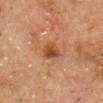This lesion was catalogued during total-body skin photography and was not selected for biopsy.
Measured at roughly 3 mm in maximum diameter.
A 15 mm crop from a total-body photograph taken for skin-cancer surveillance.
The lesion-visualizer software estimated an area of roughly 5.5 mm², a shape eccentricity near 0.6, and a symmetry-axis asymmetry near 0.2. The software also gave a border-irregularity rating of about 2/10, a within-lesion color-variation index near 4/10, and radial color variation of about 1. And it measured a classifier nevus-likeness of about 90/100 and a lesion-detection confidence of about 100/100.
From the head or neck.
A male subject, in their 60s.